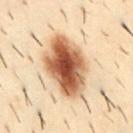biopsy status: total-body-photography surveillance lesion; no biopsy | diameter: ~7.5 mm (longest diameter) | illumination: cross-polarized illumination | site: the front of the torso | imaging modality: ~15 mm tile from a whole-body skin photo | subject: male, approximately 30 years of age | TBP lesion metrics: an outline eccentricity of about 0.75 (0 = round, 1 = elongated) and a symmetry-axis asymmetry near 0.15; a classifier nevus-likeness of about 100/100 and lesion-presence confidence of about 100/100.